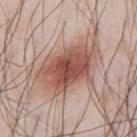Captured during whole-body skin photography for melanoma surveillance; the lesion was not biopsied.
A male patient aged around 35.
Cropped from a whole-body photographic skin survey; the tile spans about 15 mm.
Located on the chest.